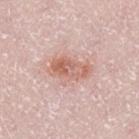From the left thigh.
The subject is a male aged 48–52.
Captured under white-light illumination.
Measured at roughly 5 mm in maximum diameter.
A lesion tile, about 15 mm wide, cut from a 3D total-body photograph.
Automated tile analysis of the lesion measured a footprint of about 11 mm² and an eccentricity of roughly 0.85.